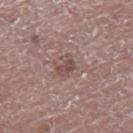Captured during whole-body skin photography for melanoma surveillance; the lesion was not biopsied. The lesion's longest dimension is about 3 mm. A lesion tile, about 15 mm wide, cut from a 3D total-body photograph. Located on the right thigh. This is a white-light tile. Automated image analysis of the tile measured a mean CIELAB color near L≈48 a*≈18 b*≈19 and a lesion-to-skin contrast of about 6.5 (normalized; higher = more distinct). A female subject, in their mid-60s.